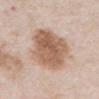Q: Is there a histopathology result?
A: catalogued during a skin exam; not biopsied
Q: How large is the lesion?
A: about 7.5 mm
Q: Automated lesion metrics?
A: a lesion area of about 27 mm², an outline eccentricity of about 0.8 (0 = round, 1 = elongated), and a symmetry-axis asymmetry near 0.2; internal color variation of about 5 on a 0–10 scale and radial color variation of about 2; a nevus-likeness score of about 90/100 and a detector confidence of about 100 out of 100 that the crop contains a lesion
Q: What lighting was used for the tile?
A: white-light
Q: What is the anatomic site?
A: the chest
Q: What is the imaging modality?
A: ~15 mm crop, total-body skin-cancer survey
Q: What are the patient's age and sex?
A: male, aged around 55